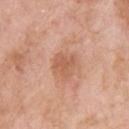Captured during whole-body skin photography for melanoma surveillance; the lesion was not biopsied.
The patient is a male roughly 60 years of age.
Imaged with white-light lighting.
Located on the back.
A roughly 15 mm field-of-view crop from a total-body skin photograph.
An algorithmic analysis of the crop reported a border-irregularity rating of about 2.5/10, a within-lesion color-variation index near 2/10, and peripheral color asymmetry of about 1. The software also gave an automated nevus-likeness rating near 0 out of 100 and a lesion-detection confidence of about 100/100.
Measured at roughly 3 mm in maximum diameter.